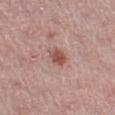biopsy status: no biopsy performed (imaged during a skin exam)
patient: female, roughly 45 years of age
acquisition: ~15 mm tile from a whole-body skin photo
automated lesion analysis: a lesion color around L≈52 a*≈24 b*≈26 in CIELAB, roughly 11 lightness units darker than nearby skin, and a lesion-to-skin contrast of about 8.5 (normalized; higher = more distinct); border irregularity of about 1.5 on a 0–10 scale, a within-lesion color-variation index near 3.5/10, and peripheral color asymmetry of about 1.5; a nevus-likeness score of about 85/100 and a lesion-detection confidence of about 100/100
lesion size: about 2.5 mm
anatomic site: the left lower leg